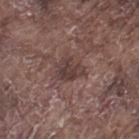Assessment:
The lesion was photographed on a routine skin check and not biopsied; there is no pathology result.
Clinical summary:
This is a white-light tile. The subject is a male aged approximately 75. Approximately 3.5 mm at its widest. A 15 mm crop from a total-body photograph taken for skin-cancer surveillance. The lesion-visualizer software estimated a footprint of about 7 mm², an eccentricity of roughly 0.6, and a symmetry-axis asymmetry near 0.3. The software also gave a normalized lesion–skin contrast near 7.5. The analysis additionally found a border-irregularity index near 3.5/10, a within-lesion color-variation index near 3/10, and peripheral color asymmetry of about 1. The software also gave a detector confidence of about 90 out of 100 that the crop contains a lesion. The lesion is on the left thigh.